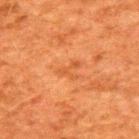Clinical impression:
The lesion was tiled from a total-body skin photograph and was not biopsied.
Background:
The recorded lesion diameter is about 2.5 mm. The lesion is on the back. Cropped from a total-body skin-imaging series; the visible field is about 15 mm. The patient is a male approximately 60 years of age. Captured under cross-polarized illumination.